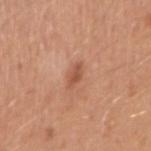<tbp_lesion>
  <biopsy_status>not biopsied; imaged during a skin examination</biopsy_status>
  <lighting>white-light</lighting>
  <image>
    <source>total-body photography crop</source>
    <field_of_view_mm>15</field_of_view_mm>
  </image>
  <site>arm</site>
  <patient>
    <sex>male</sex>
    <age_approx>40</age_approx>
  </patient>
</tbp_lesion>